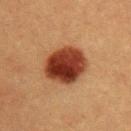A male subject, aged around 40. This is a cross-polarized tile. A close-up tile cropped from a whole-body skin photograph, about 15 mm across. On the left upper arm.